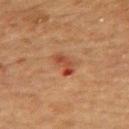Imaged with cross-polarized lighting.
The subject is a female aged 68 to 72.
A region of skin cropped from a whole-body photographic capture, roughly 15 mm wide.
The recorded lesion diameter is about 3 mm.
On the upper back.
An algorithmic analysis of the crop reported a lesion color around L≈39 a*≈25 b*≈30 in CIELAB and a lesion-to-skin contrast of about 8 (normalized; higher = more distinct). The analysis additionally found a classifier nevus-likeness of about 0/100 and lesion-presence confidence of about 100/100.